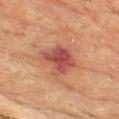Q: Who is the patient?
A: female, aged approximately 80
Q: Where on the body is the lesion?
A: the chest
Q: What lighting was used for the tile?
A: cross-polarized illumination
Q: How large is the lesion?
A: ~4.5 mm (longest diameter)
Q: What is the imaging modality?
A: ~15 mm crop, total-body skin-cancer survey